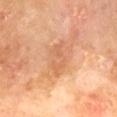<record>
  <biopsy_status>not biopsied; imaged during a skin examination</biopsy_status>
  <lighting>cross-polarized</lighting>
  <site>back</site>
  <image>
    <source>total-body photography crop</source>
    <field_of_view_mm>15</field_of_view_mm>
  </image>
  <patient>
    <sex>male</sex>
    <age_approx>70</age_approx>
  </patient>
</record>